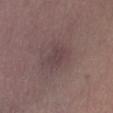Assessment:
Captured during whole-body skin photography for melanoma surveillance; the lesion was not biopsied.
Acquisition and patient details:
A close-up tile cropped from a whole-body skin photograph, about 15 mm across. On the right lower leg. Automated tile analysis of the lesion measured a footprint of about 4.5 mm² and two-axis asymmetry of about 0.35. It also reported a lesion color around L≈41 a*≈17 b*≈14 in CIELAB and a normalized border contrast of about 5. Approximately 3 mm at its widest. Imaged with white-light lighting. A female subject, aged 43–47.